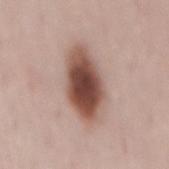notes: imaged on a skin check; not biopsied | image: ~15 mm tile from a whole-body skin photo | automated metrics: a border-irregularity index near 2/10 | site: the mid back | subject: female, in their 50s | lighting: white-light.